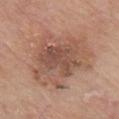This lesion was catalogued during total-body skin photography and was not selected for biopsy.
The total-body-photography lesion software estimated a lesion color around L≈53 a*≈20 b*≈28 in CIELAB, roughly 9 lightness units darker than nearby skin, and a lesion-to-skin contrast of about 6.5 (normalized; higher = more distinct).
A 15 mm crop from a total-body photograph taken for skin-cancer surveillance.
The lesion is on the chest.
The subject is a male in their 80s.